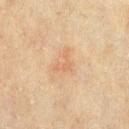Findings:
– biopsy status — catalogued during a skin exam; not biopsied
– lesion size — ~3 mm (longest diameter)
– image — ~15 mm tile from a whole-body skin photo
– anatomic site — the chest
– image-analysis metrics — border irregularity of about 8 on a 0–10 scale and a peripheral color-asymmetry measure near 0; a classifier nevus-likeness of about 0/100
– patient — male, aged 68 to 72